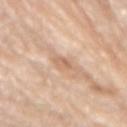Q: Patient demographics?
A: male, in their 80s
Q: Lesion size?
A: ~2.5 mm (longest diameter)
Q: What did automated image analysis measure?
A: a nevus-likeness score of about 0/100 and a lesion-detection confidence of about 90/100
Q: What lighting was used for the tile?
A: white-light illumination
Q: Where on the body is the lesion?
A: the lower back
Q: What kind of image is this?
A: ~15 mm crop, total-body skin-cancer survey